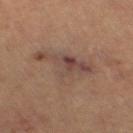Clinical impression: Recorded during total-body skin imaging; not selected for excision or biopsy. Image and clinical context: Approximately 7 mm at its widest. The patient is a female about 65 years old. On the right thigh. This image is a 15 mm lesion crop taken from a total-body photograph. This is a cross-polarized tile. Automated tile analysis of the lesion measured a lesion area of about 15 mm², an eccentricity of roughly 0.85, and two-axis asymmetry of about 0.5. The analysis additionally found a lesion color around L≈42 a*≈16 b*≈23 in CIELAB, about 7 CIELAB-L* units darker than the surrounding skin, and a normalized border contrast of about 6.5. The software also gave a border-irregularity rating of about 6.5/10, a color-variation rating of about 6.5/10, and radial color variation of about 2. The analysis additionally found a classifier nevus-likeness of about 0/100 and a lesion-detection confidence of about 80/100.